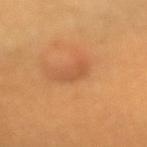No biopsy was performed on this lesion — it was imaged during a full skin examination and was not determined to be concerning. A roughly 15 mm field-of-view crop from a total-body skin photograph. This is a cross-polarized tile. From the arm. A female patient aged around 25. Approximately 3.5 mm at its widest.